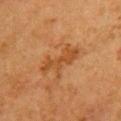Context:
From the chest. A roughly 15 mm field-of-view crop from a total-body skin photograph. Captured under cross-polarized illumination. An algorithmic analysis of the crop reported about 7 CIELAB-L* units darker than the surrounding skin and a normalized lesion–skin contrast near 6.5. And it measured border irregularity of about 6.5 on a 0–10 scale, a within-lesion color-variation index near 1/10, and radial color variation of about 0.5. A female subject approximately 50 years of age.